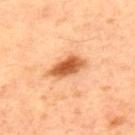Q: Was a biopsy performed?
A: catalogued during a skin exam; not biopsied
Q: How was the tile lit?
A: cross-polarized illumination
Q: What kind of image is this?
A: ~15 mm tile from a whole-body skin photo
Q: Where on the body is the lesion?
A: the upper back
Q: What did automated image analysis measure?
A: an average lesion color of about L≈59 a*≈29 b*≈42 (CIELAB) and a lesion–skin lightness drop of about 17
Q: How large is the lesion?
A: ~4.5 mm (longest diameter)
Q: What are the patient's age and sex?
A: male, aged approximately 60